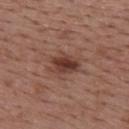follow-up = catalogued during a skin exam; not biopsied
location = the upper back
subject = female, aged around 50
size = ~4 mm (longest diameter)
image source = total-body-photography crop, ~15 mm field of view